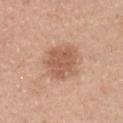The lesion was photographed on a routine skin check and not biopsied; there is no pathology result.
A 15 mm close-up tile from a total-body photography series done for melanoma screening.
This is a white-light tile.
A female patient, about 30 years old.
The recorded lesion diameter is about 4 mm.
From the front of the torso.